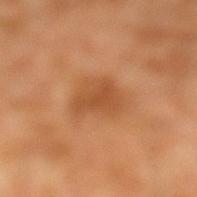{"image": {"source": "total-body photography crop", "field_of_view_mm": 15}, "patient": {"sex": "male", "age_approx": 30}, "lighting": "cross-polarized", "site": "left lower leg", "automated_metrics": {"eccentricity": 0.6}}